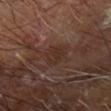Q: Was a biopsy performed?
A: catalogued during a skin exam; not biopsied
Q: How large is the lesion?
A: about 3 mm
Q: How was this image acquired?
A: total-body-photography crop, ~15 mm field of view
Q: Patient demographics?
A: male, aged 63 to 67
Q: What is the anatomic site?
A: the right forearm
Q: Automated lesion metrics?
A: a footprint of about 5 mm², a shape eccentricity near 0.65, and a symmetry-axis asymmetry near 0.25; a border-irregularity rating of about 2.5/10, a within-lesion color-variation index near 1.5/10, and peripheral color asymmetry of about 0.5
Q: How was the tile lit?
A: cross-polarized illumination